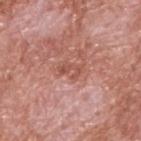This lesion was catalogued during total-body skin photography and was not selected for biopsy. Located on the upper back. The subject is a male roughly 60 years of age. A roughly 15 mm field-of-view crop from a total-body skin photograph. About 3 mm across.